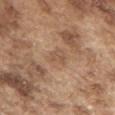The lesion was photographed on a routine skin check and not biopsied; there is no pathology result. On the back. Imaged with white-light lighting. A lesion tile, about 15 mm wide, cut from a 3D total-body photograph. A male patient aged around 75. Longest diameter approximately 2.5 mm.